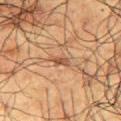Q: Was a biopsy performed?
A: imaged on a skin check; not biopsied
Q: Lesion location?
A: the right upper arm
Q: What kind of image is this?
A: ~15 mm crop, total-body skin-cancer survey
Q: Patient demographics?
A: male, roughly 60 years of age